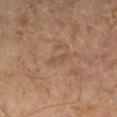| key | value |
|---|---|
| workup | no biopsy performed (imaged during a skin exam) |
| lesion diameter | about 2.5 mm |
| patient | male, aged around 55 |
| image | total-body-photography crop, ~15 mm field of view |
| TBP lesion metrics | a color-variation rating of about 0/10 and radial color variation of about 0; an automated nevus-likeness rating near 0 out of 100 and a detector confidence of about 100 out of 100 that the crop contains a lesion |
| location | the left lower leg |
| lighting | cross-polarized |